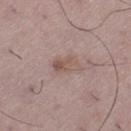imaging modality: ~15 mm crop, total-body skin-cancer survey
location: the left thigh
TBP lesion metrics: a border-irregularity rating of about 2.5/10 and peripheral color asymmetry of about 2.5; an automated nevus-likeness rating near 10 out of 100 and lesion-presence confidence of about 100/100
patient: male, in their mid-50s
lighting: white-light
size: ~3 mm (longest diameter)